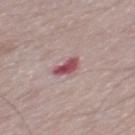workup = imaged on a skin check; not biopsied
subject = male, aged approximately 65
automated lesion analysis = a lesion color around L≈50 a*≈28 b*≈17 in CIELAB and a normalized border contrast of about 10; lesion-presence confidence of about 100/100
image = ~15 mm tile from a whole-body skin photo
anatomic site = the mid back
illumination = white-light illumination
lesion diameter = ≈3 mm